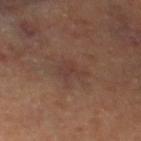The lesion was photographed on a routine skin check and not biopsied; there is no pathology result.
From the left thigh.
Captured under cross-polarized illumination.
Automated tile analysis of the lesion measured an eccentricity of roughly 0.8. The analysis additionally found border irregularity of about 5 on a 0–10 scale, internal color variation of about 2.5 on a 0–10 scale, and radial color variation of about 1.
A 15 mm close-up extracted from a 3D total-body photography capture.
The subject is a male aged around 60.
The recorded lesion diameter is about 3.5 mm.